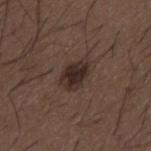Recorded during total-body skin imaging; not selected for excision or biopsy. From the mid back. A region of skin cropped from a whole-body photographic capture, roughly 15 mm wide. A male subject approximately 50 years of age.